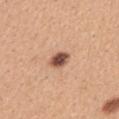Captured during whole-body skin photography for melanoma surveillance; the lesion was not biopsied.
A 15 mm crop from a total-body photograph taken for skin-cancer surveillance.
On the back.
A female patient roughly 30 years of age.
The lesion-visualizer software estimated an average lesion color of about L≈52 a*≈22 b*≈29 (CIELAB) and a lesion-to-skin contrast of about 12 (normalized; higher = more distinct). The software also gave a border-irregularity index near 2/10, a within-lesion color-variation index near 5.5/10, and a peripheral color-asymmetry measure near 1.5.
About 3 mm across.